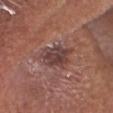The subject is a male aged 63–67. Approximately 4 mm at its widest. A 15 mm close-up extracted from a 3D total-body photography capture. The total-body-photography lesion software estimated a mean CIELAB color near L≈41 a*≈20 b*≈20, roughly 10 lightness units darker than nearby skin, and a lesion-to-skin contrast of about 8.5 (normalized; higher = more distinct). The lesion is on the head or neck.